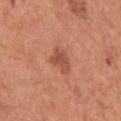notes: catalogued during a skin exam; not biopsied | tile lighting: white-light illumination | size: about 3 mm | imaging modality: total-body-photography crop, ~15 mm field of view | image-analysis metrics: an average lesion color of about L≈52 a*≈27 b*≈33 (CIELAB), a lesion–skin lightness drop of about 9, and a normalized border contrast of about 6.5; a color-variation rating of about 2/10 and a peripheral color-asymmetry measure near 0.5; a nevus-likeness score of about 70/100 and a detector confidence of about 100 out of 100 that the crop contains a lesion | subject: male, aged 63 to 67 | location: the chest.